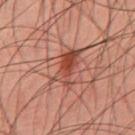lesion diameter = ~5 mm (longest diameter)
image-analysis metrics = an area of roughly 8.5 mm² and a shape eccentricity near 0.9; a border-irregularity index near 5/10, internal color variation of about 6.5 on a 0–10 scale, and radial color variation of about 2
anatomic site = the back
image source = 15 mm crop, total-body photography
lighting = cross-polarized illumination
subject = male, approximately 45 years of age